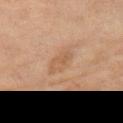Assessment:
Captured during whole-body skin photography for melanoma surveillance; the lesion was not biopsied.
Context:
About 5 mm across. Captured under cross-polarized illumination. The lesion is on the right thigh. A region of skin cropped from a whole-body photographic capture, roughly 15 mm wide. The patient is a female aged 68–72.